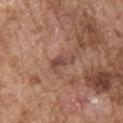notes=catalogued during a skin exam; not biopsied | illumination=white-light | image source=total-body-photography crop, ~15 mm field of view | TBP lesion metrics=a border-irregularity rating of about 4.5/10 and a within-lesion color-variation index near 1/10; an automated nevus-likeness rating near 0 out of 100 and lesion-presence confidence of about 100/100 | location=the right upper arm | lesion diameter=~3 mm (longest diameter) | subject=male, in their mid- to late 70s.